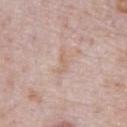The lesion was photographed on a routine skin check and not biopsied; there is no pathology result. A male subject aged 68–72. The lesion is located on the abdomen. A lesion tile, about 15 mm wide, cut from a 3D total-body photograph.